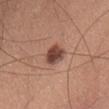Assessment:
Recorded during total-body skin imaging; not selected for excision or biopsy.
Background:
The recorded lesion diameter is about 3.5 mm. A close-up tile cropped from a whole-body skin photograph, about 15 mm across. A male patient aged approximately 60. Located on the chest. Automated tile analysis of the lesion measured a mean CIELAB color near L≈45 a*≈22 b*≈26 and roughly 14 lightness units darker than nearby skin. The software also gave a border-irregularity index near 1.5/10, a within-lesion color-variation index near 4.5/10, and radial color variation of about 1.5. And it measured a nevus-likeness score of about 95/100.